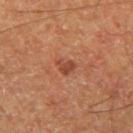The lesion was photographed on a routine skin check and not biopsied; there is no pathology result.
The lesion-visualizer software estimated a mean CIELAB color near L≈40 a*≈23 b*≈29, a lesion–skin lightness drop of about 8, and a normalized border contrast of about 7. The software also gave internal color variation of about 1 on a 0–10 scale and a peripheral color-asymmetry measure near 0.5. And it measured an automated nevus-likeness rating near 25 out of 100.
A male patient approximately 60 years of age.
A 15 mm close-up tile from a total-body photography series done for melanoma screening.
The lesion's longest dimension is about 2.5 mm.
This is a cross-polarized tile.